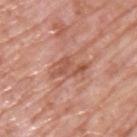Part of a total-body skin-imaging series; this lesion was reviewed on a skin check and was not flagged for biopsy.
A close-up tile cropped from a whole-body skin photograph, about 15 mm across.
Imaged with white-light lighting.
The total-body-photography lesion software estimated a footprint of about 7.5 mm², a shape eccentricity near 0.9, and a symmetry-axis asymmetry near 0.3. It also reported a mean CIELAB color near L≈55 a*≈25 b*≈31, a lesion–skin lightness drop of about 9, and a normalized lesion–skin contrast near 6.5.
The recorded lesion diameter is about 5 mm.
From the upper back.
A male patient, about 70 years old.